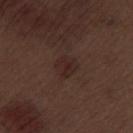Background:
Approximately 3 mm at its widest. Captured under white-light illumination. Cropped from a total-body skin-imaging series; the visible field is about 15 mm. On the left thigh. The subject is a male approximately 70 years of age.